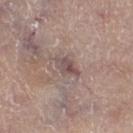Clinical impression:
Recorded during total-body skin imaging; not selected for excision or biopsy.
Acquisition and patient details:
This is a white-light tile. The lesion's longest dimension is about 3.5 mm. The lesion-visualizer software estimated an area of roughly 4.5 mm², an outline eccentricity of about 0.85 (0 = round, 1 = elongated), and two-axis asymmetry of about 0.3. It also reported a lesion–skin lightness drop of about 10. And it measured an automated nevus-likeness rating near 0 out of 100 and a lesion-detection confidence of about 55/100. A 15 mm close-up tile from a total-body photography series done for melanoma screening. A male patient aged 78 to 82. On the right thigh.